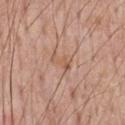Impression:
Captured during whole-body skin photography for melanoma surveillance; the lesion was not biopsied.
Acquisition and patient details:
Imaged with white-light lighting. The lesion is located on the chest. Automated image analysis of the tile measured a footprint of about 4 mm² and two-axis asymmetry of about 0.4. The analysis additionally found a mean CIELAB color near L≈57 a*≈20 b*≈30 and roughly 7 lightness units darker than nearby skin. And it measured an automated nevus-likeness rating near 0 out of 100. Measured at roughly 3 mm in maximum diameter. A lesion tile, about 15 mm wide, cut from a 3D total-body photograph. The subject is a male in their mid- to late 50s.